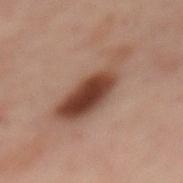A lesion tile, about 15 mm wide, cut from a 3D total-body photograph.
The recorded lesion diameter is about 8 mm.
Captured under cross-polarized illumination.
A female subject, aged around 55.
Automated image analysis of the tile measured a footprint of about 18 mm², an outline eccentricity of about 0.95 (0 = round, 1 = elongated), and a symmetry-axis asymmetry near 0.35.
The lesion is on the mid back.